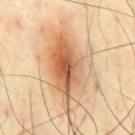This lesion was catalogued during total-body skin photography and was not selected for biopsy.
A lesion tile, about 15 mm wide, cut from a 3D total-body photograph.
The patient is a male aged 63–67.
From the abdomen.
Imaged with cross-polarized lighting.